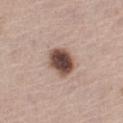Captured during whole-body skin photography for melanoma surveillance; the lesion was not biopsied. A 15 mm crop from a total-body photograph taken for skin-cancer surveillance. A male patient approximately 65 years of age. The total-body-photography lesion software estimated a footprint of about 9.5 mm² and two-axis asymmetry of about 0.15. It also reported a lesion–skin lightness drop of about 20 and a normalized border contrast of about 13.5. The software also gave a classifier nevus-likeness of about 75/100. The tile uses white-light illumination. Located on the front of the torso. The lesion's longest dimension is about 4 mm.